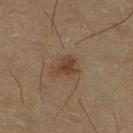biopsy_status: not biopsied; imaged during a skin examination
lighting: cross-polarized
image:
  source: total-body photography crop
  field_of_view_mm: 15
site: right lower leg
lesion_size:
  long_diameter_mm_approx: 3.0
patient:
  sex: female
  age_approx: 70